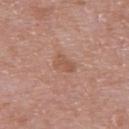Automated image analysis of the tile measured a color-variation rating of about 1.5/10 and radial color variation of about 0.5. And it measured a nevus-likeness score of about 0/100. A roughly 15 mm field-of-view crop from a total-body skin photograph. The lesion is located on the upper back. A female patient, aged approximately 30. Captured under white-light illumination.